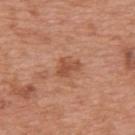Imaged during a routine full-body skin examination; the lesion was not biopsied and no histopathology is available. Located on the upper back. Captured under white-light illumination. Cropped from a total-body skin-imaging series; the visible field is about 15 mm. The subject is a male about 70 years old. Longest diameter approximately 3 mm.